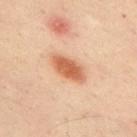automated metrics: an area of roughly 7.5 mm², an outline eccentricity of about 0.9 (0 = round, 1 = elongated), and a shape-asymmetry score of about 0.2 (0 = symmetric); border irregularity of about 2 on a 0–10 scale, a within-lesion color-variation index near 2.5/10, and radial color variation of about 0.5; an automated nevus-likeness rating near 100 out of 100 and a lesion-detection confidence of about 100/100
diameter: ≈4.5 mm
acquisition: ~15 mm tile from a whole-body skin photo
illumination: cross-polarized illumination
anatomic site: the back
patient: male, aged 48–52